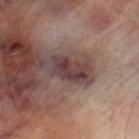Recorded during total-body skin imaging; not selected for excision or biopsy. On the left thigh. The recorded lesion diameter is about 4 mm. Cropped from a total-body skin-imaging series; the visible field is about 15 mm. Automated image analysis of the tile measured a border-irregularity rating of about 3/10, a within-lesion color-variation index near 5/10, and peripheral color asymmetry of about 1.5. A male patient, aged approximately 70. Captured under cross-polarized illumination.